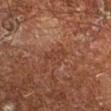The lesion was photographed on a routine skin check and not biopsied; there is no pathology result.